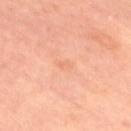| field | value |
|---|---|
| follow-up | no biopsy performed (imaged during a skin exam) |
| image source | ~15 mm tile from a whole-body skin photo |
| subject | male, aged 68–72 |
| site | the right thigh |